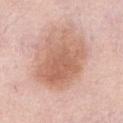follow-up: imaged on a skin check; not biopsied
anatomic site: the abdomen
patient: female, aged around 50
image: ~15 mm tile from a whole-body skin photo